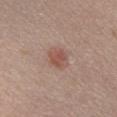follow-up = total-body-photography surveillance lesion; no biopsy | location = the chest | image source = total-body-photography crop, ~15 mm field of view | subject = female, in their mid- to late 50s.